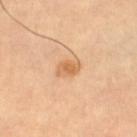The lesion was photographed on a routine skin check and not biopsied; there is no pathology result.
Imaged with cross-polarized lighting.
A female subject aged approximately 70.
The lesion is located on the left thigh.
The lesion's longest dimension is about 3 mm.
Cropped from a total-body skin-imaging series; the visible field is about 15 mm.